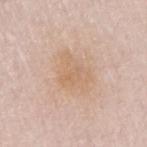biopsy status — total-body-photography surveillance lesion; no biopsy
location — the arm
tile lighting — white-light
acquisition — ~15 mm tile from a whole-body skin photo
lesion diameter — about 4.5 mm
subject — male, approximately 65 years of age